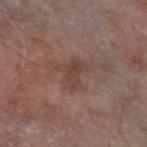<case>
  <biopsy_status>not biopsied; imaged during a skin examination</biopsy_status>
  <site>arm</site>
  <patient>
    <sex>male</sex>
    <age_approx>60</age_approx>
  </patient>
  <image>
    <source>total-body photography crop</source>
    <field_of_view_mm>15</field_of_view_mm>
  </image>
</case>